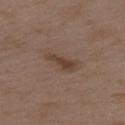Recorded during total-body skin imaging; not selected for excision or biopsy. A region of skin cropped from a whole-body photographic capture, roughly 15 mm wide. From the left upper arm. A female subject, aged 33 to 37. This is a white-light tile.